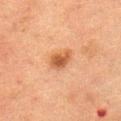Part of a total-body skin-imaging series; this lesion was reviewed on a skin check and was not flagged for biopsy. The lesion is located on the abdomen. Measured at roughly 3 mm in maximum diameter. A female subject, aged approximately 50. Cropped from a total-body skin-imaging series; the visible field is about 15 mm.